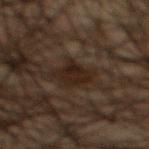The lesion was tiled from a total-body skin photograph and was not biopsied. The lesion-visualizer software estimated an automated nevus-likeness rating near 5 out of 100 and a detector confidence of about 95 out of 100 that the crop contains a lesion. Approximately 3.5 mm at its widest. A male patient aged around 50. Imaged with cross-polarized lighting. On the mid back. A roughly 15 mm field-of-view crop from a total-body skin photograph.